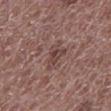A close-up tile cropped from a whole-body skin photograph, about 15 mm across.
Approximately 3 mm at its widest.
A male subject about 70 years old.
On the left lower leg.
The tile uses white-light illumination.
Automated image analysis of the tile measured a lesion area of about 5 mm² and two-axis asymmetry of about 0.55. The analysis additionally found border irregularity of about 5.5 on a 0–10 scale. The analysis additionally found a nevus-likeness score of about 0/100 and a lesion-detection confidence of about 95/100.